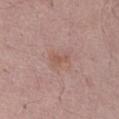Clinical impression: Part of a total-body skin-imaging series; this lesion was reviewed on a skin check and was not flagged for biopsy. Acquisition and patient details: Measured at roughly 2.5 mm in maximum diameter. The lesion is located on the abdomen. A male subject aged 68 to 72. The tile uses white-light illumination. A close-up tile cropped from a whole-body skin photograph, about 15 mm across.